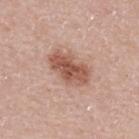Imaged during a routine full-body skin examination; the lesion was not biopsied and no histopathology is available. A 15 mm crop from a total-body photograph taken for skin-cancer surveillance. The lesion's longest dimension is about 5 mm. This is a white-light tile. The lesion-visualizer software estimated a footprint of about 12 mm² and a shape eccentricity near 0.85. The analysis additionally found a lesion color around L≈54 a*≈23 b*≈28 in CIELAB. The analysis additionally found an automated nevus-likeness rating near 65 out of 100 and lesion-presence confidence of about 100/100. From the upper back. The subject is a female roughly 40 years of age.